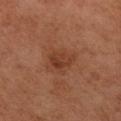The lesion was photographed on a routine skin check and not biopsied; there is no pathology result.
A female patient aged around 60.
Located on the arm.
The total-body-photography lesion software estimated a footprint of about 5 mm², an outline eccentricity of about 0.7 (0 = round, 1 = elongated), and a symmetry-axis asymmetry near 0.3.
Approximately 3 mm at its widest.
The tile uses cross-polarized illumination.
Cropped from a total-body skin-imaging series; the visible field is about 15 mm.